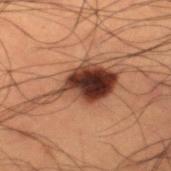This lesion was catalogued during total-body skin photography and was not selected for biopsy. A 15 mm close-up tile from a total-body photography series done for melanoma screening. A male subject, roughly 50 years of age. The lesion-visualizer software estimated a border-irregularity rating of about 6/10 and peripheral color asymmetry of about 3. The software also gave a nevus-likeness score of about 20/100 and a detector confidence of about 100 out of 100 that the crop contains a lesion. Imaged with cross-polarized lighting. Located on the right lower leg. The lesion's longest dimension is about 8 mm.